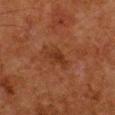| field | value |
|---|---|
| workup | imaged on a skin check; not biopsied |